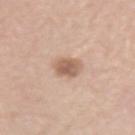<tbp_lesion>
<biopsy_status>not biopsied; imaged during a skin examination</biopsy_status>
<image>
  <source>total-body photography crop</source>
  <field_of_view_mm>15</field_of_view_mm>
</image>
<patient>
  <sex>female</sex>
  <age_approx>70</age_approx>
</patient>
<site>left upper arm</site>
</tbp_lesion>